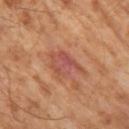<tbp_lesion>
  <biopsy_status>not biopsied; imaged during a skin examination</biopsy_status>
  <lesion_size>
    <long_diameter_mm_approx>4.5</long_diameter_mm_approx>
  </lesion_size>
  <patient>
    <sex>male</sex>
    <age_approx>65</age_approx>
  </patient>
  <image>
    <source>total-body photography crop</source>
    <field_of_view_mm>15</field_of_view_mm>
  </image>
  <lighting>cross-polarized</lighting>
</tbp_lesion>